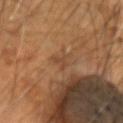biopsy status: imaged on a skin check; not biopsied | imaging modality: ~15 mm crop, total-body skin-cancer survey | subject: male, approximately 55 years of age | site: the head or neck | diameter: ≈3 mm | lighting: cross-polarized illumination | automated lesion analysis: a symmetry-axis asymmetry near 0.55; a border-irregularity rating of about 6/10 and peripheral color asymmetry of about 0.